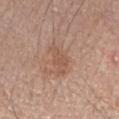Recorded during total-body skin imaging; not selected for excision or biopsy.
Automated image analysis of the tile measured an average lesion color of about L≈54 a*≈20 b*≈29 (CIELAB), roughly 7 lightness units darker than nearby skin, and a normalized lesion–skin contrast near 5.5. The analysis additionally found border irregularity of about 5 on a 0–10 scale, a color-variation rating of about 1.5/10, and peripheral color asymmetry of about 0.5.
The lesion's longest dimension is about 4 mm.
Imaged with white-light lighting.
A male patient roughly 60 years of age.
A 15 mm close-up extracted from a 3D total-body photography capture.
The lesion is located on the right forearm.